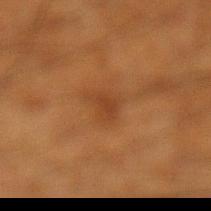Imaged during a routine full-body skin examination; the lesion was not biopsied and no histopathology is available. Located on the left lower leg. A male subject, roughly 60 years of age. The recorded lesion diameter is about 3 mm. Cropped from a total-body skin-imaging series; the visible field is about 15 mm. The total-body-photography lesion software estimated an average lesion color of about L≈35 a*≈21 b*≈32 (CIELAB) and roughly 6 lightness units darker than nearby skin. The analysis additionally found a classifier nevus-likeness of about 5/100 and a detector confidence of about 100 out of 100 that the crop contains a lesion.